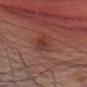The lesion was photographed on a routine skin check and not biopsied; there is no pathology result. The lesion is located on the head or neck. Approximately 4 mm at its widest. Automated tile analysis of the lesion measured border irregularity of about 2.5 on a 0–10 scale, internal color variation of about 5 on a 0–10 scale, and radial color variation of about 1.5. It also reported a nevus-likeness score of about 5/100. A 15 mm crop from a total-body photograph taken for skin-cancer surveillance. The subject is a female aged 38–42. Captured under white-light illumination.